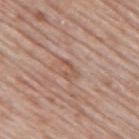This image is a 15 mm lesion crop taken from a total-body photograph. Approximately 2.5 mm at its widest. A male patient roughly 60 years of age. This is a white-light tile. On the upper back.